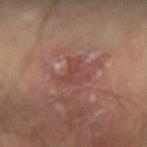The lesion was tiled from a total-body skin photograph and was not biopsied. The patient is a male aged around 70. This image is a 15 mm lesion crop taken from a total-body photograph. Captured under cross-polarized illumination. From the left forearm. Approximately 3 mm at its widest.